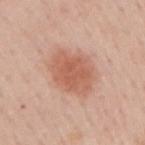This lesion was catalogued during total-body skin photography and was not selected for biopsy.
Imaged with white-light lighting.
A male patient, aged 58 to 62.
Cropped from a total-body skin-imaging series; the visible field is about 15 mm.
The lesion is on the right upper arm.
The recorded lesion diameter is about 5 mm.